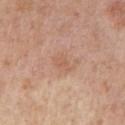biopsy_status: not biopsied; imaged during a skin examination
image:
  source: total-body photography crop
  field_of_view_mm: 15
lighting: white-light
site: left upper arm
lesion_size:
  long_diameter_mm_approx: 2.5
patient:
  sex: female
  age_approx: 60